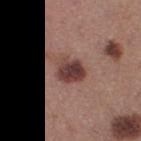Q: Was a biopsy performed?
A: no biopsy performed (imaged during a skin exam)
Q: Automated lesion metrics?
A: a footprint of about 8.5 mm², a shape eccentricity near 0.65, and a symmetry-axis asymmetry near 0.3; a border-irregularity rating of about 2.5/10 and peripheral color asymmetry of about 2.5; a nevus-likeness score of about 85/100 and lesion-presence confidence of about 100/100
Q: What kind of image is this?
A: ~15 mm tile from a whole-body skin photo
Q: Lesion location?
A: the right thigh
Q: What is the lesion's diameter?
A: ≈4 mm
Q: Patient demographics?
A: female, in their mid- to late 30s
Q: Illumination type?
A: white-light